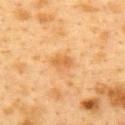A region of skin cropped from a whole-body photographic capture, roughly 15 mm wide. A female patient, aged 38 to 42. On the upper back. Measured at roughly 2.5 mm in maximum diameter. This is a cross-polarized tile.